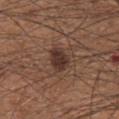biopsy status: imaged on a skin check; not biopsied
subject: male, aged approximately 65
lighting: white-light
acquisition: ~15 mm tile from a whole-body skin photo
automated metrics: an area of roughly 6 mm², an eccentricity of roughly 0.7, and a shape-asymmetry score of about 0.15 (0 = symmetric); internal color variation of about 2.5 on a 0–10 scale and radial color variation of about 1; a classifier nevus-likeness of about 85/100 and a detector confidence of about 100 out of 100 that the crop contains a lesion
location: the abdomen
lesion size: ≈3 mm